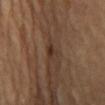Assessment: This lesion was catalogued during total-body skin photography and was not selected for biopsy. Context: The recorded lesion diameter is about 3 mm. On the abdomen. A female subject, about 70 years old. An algorithmic analysis of the crop reported a lesion area of about 3.5 mm², an outline eccentricity of about 0.85 (0 = round, 1 = elongated), and a shape-asymmetry score of about 0.35 (0 = symmetric). And it measured an average lesion color of about L≈31 a*≈16 b*≈24 (CIELAB), about 7 CIELAB-L* units darker than the surrounding skin, and a lesion-to-skin contrast of about 7 (normalized; higher = more distinct). The analysis additionally found a border-irregularity rating of about 3.5/10 and peripheral color asymmetry of about 1. It also reported lesion-presence confidence of about 90/100. A close-up tile cropped from a whole-body skin photograph, about 15 mm across.